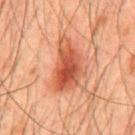| key | value |
|---|---|
| workup | total-body-photography surveillance lesion; no biopsy |
| size | about 6.5 mm |
| location | the mid back |
| lighting | cross-polarized illumination |
| image | 15 mm crop, total-body photography |
| automated metrics | a nevus-likeness score of about 100/100 |
| patient | male, approximately 45 years of age |